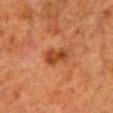notes: no biopsy performed (imaged during a skin exam) | lesion diameter: about 3 mm | subject: female, aged around 55 | tile lighting: cross-polarized | anatomic site: the head or neck | imaging modality: ~15 mm crop, total-body skin-cancer survey | TBP lesion metrics: a mean CIELAB color near L≈37 a*≈26 b*≈34, a lesion–skin lightness drop of about 10, and a normalized border contrast of about 8.5.